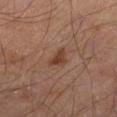Context: A lesion tile, about 15 mm wide, cut from a 3D total-body photograph. Measured at roughly 2.5 mm in maximum diameter. From the left thigh. Automated image analysis of the tile measured an outline eccentricity of about 0.55 (0 = round, 1 = elongated) and two-axis asymmetry of about 0.45. And it measured a border-irregularity rating of about 4.5/10 and a peripheral color-asymmetry measure near 0.5. The analysis additionally found an automated nevus-likeness rating near 80 out of 100 and a lesion-detection confidence of about 100/100. The subject is a male aged 53–57.